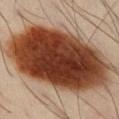Q: Was a biopsy performed?
A: no biopsy performed (imaged during a skin exam)